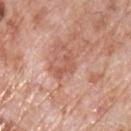| field | value |
|---|---|
| biopsy status | catalogued during a skin exam; not biopsied |
| lesion diameter | ~3.5 mm (longest diameter) |
| body site | the chest |
| subject | male, in their 70s |
| automated lesion analysis | a lesion color around L≈57 a*≈25 b*≈29 in CIELAB, about 8 CIELAB-L* units darker than the surrounding skin, and a normalized lesion–skin contrast near 6; border irregularity of about 5.5 on a 0–10 scale, internal color variation of about 1 on a 0–10 scale, and radial color variation of about 0; a nevus-likeness score of about 0/100 and a lesion-detection confidence of about 100/100 |
| acquisition | 15 mm crop, total-body photography |